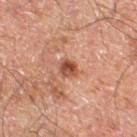The patient is a male aged 58 to 62.
The lesion is on the right thigh.
A lesion tile, about 15 mm wide, cut from a 3D total-body photograph.
The lesion-visualizer software estimated a lesion area of about 3.5 mm², an outline eccentricity of about 0.2 (0 = round, 1 = elongated), and a symmetry-axis asymmetry near 0.25.